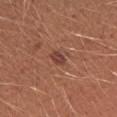• patient: female, aged 23 to 27
• illumination: white-light
• size: ≈2.5 mm
• image-analysis metrics: a footprint of about 3 mm² and a shape-asymmetry score of about 0.2 (0 = symmetric); an average lesion color of about L≈42 a*≈25 b*≈26 (CIELAB) and roughly 9 lightness units darker than nearby skin
• image: 15 mm crop, total-body photography
• body site: the right forearm